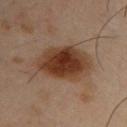Assessment:
Recorded during total-body skin imaging; not selected for excision or biopsy.